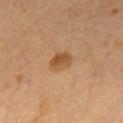Acquisition and patient details: A male patient, in their mid- to late 50s. Measured at roughly 3 mm in maximum diameter. Automated tile analysis of the lesion measured a lesion area of about 5.5 mm², an eccentricity of roughly 0.6, and a shape-asymmetry score of about 0.15 (0 = symmetric). And it measured border irregularity of about 1.5 on a 0–10 scale, internal color variation of about 3 on a 0–10 scale, and radial color variation of about 1. The analysis additionally found an automated nevus-likeness rating near 85 out of 100 and a detector confidence of about 100 out of 100 that the crop contains a lesion. On the back. A roughly 15 mm field-of-view crop from a total-body skin photograph.